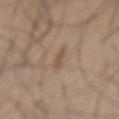This lesion was catalogued during total-body skin photography and was not selected for biopsy. A male patient, in their mid-30s. A close-up tile cropped from a whole-body skin photograph, about 15 mm across. From the mid back. This is a white-light tile.